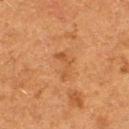Notes:
– workup — total-body-photography surveillance lesion; no biopsy
– tile lighting — cross-polarized
– acquisition — ~15 mm tile from a whole-body skin photo
– diameter — about 3.5 mm
– site — the left thigh
– subject — female, aged around 50
– image-analysis metrics — a lesion area of about 4 mm² and a shape eccentricity near 0.9; a border-irregularity rating of about 8/10, internal color variation of about 0.5 on a 0–10 scale, and radial color variation of about 0; a nevus-likeness score of about 0/100 and lesion-presence confidence of about 100/100